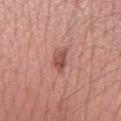follow-up=catalogued during a skin exam; not biopsied
patient=male, aged around 60
body site=the left forearm
illumination=white-light
image=15 mm crop, total-body photography
lesion diameter=≈3 mm